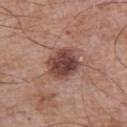workup = total-body-photography surveillance lesion; no biopsy | patient = male, in their mid- to late 60s | tile lighting = white-light illumination | site = the left upper arm | automated lesion analysis = a lesion color around L≈43 a*≈21 b*≈24 in CIELAB and a normalized lesion–skin contrast near 11; border irregularity of about 1.5 on a 0–10 scale, internal color variation of about 5 on a 0–10 scale, and radial color variation of about 1.5; a nevus-likeness score of about 60/100 and a detector confidence of about 100 out of 100 that the crop contains a lesion | size = ~4 mm (longest diameter) | image = total-body-photography crop, ~15 mm field of view.